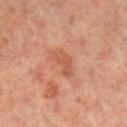This lesion was catalogued during total-body skin photography and was not selected for biopsy. A female subject aged around 60. A roughly 15 mm field-of-view crop from a total-body skin photograph. On the leg.